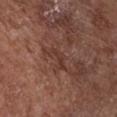This lesion was catalogued during total-body skin photography and was not selected for biopsy. A roughly 15 mm field-of-view crop from a total-body skin photograph. The total-body-photography lesion software estimated an area of roughly 3.5 mm² and an outline eccentricity of about 0.9 (0 = round, 1 = elongated). The lesion is located on the chest. A female subject aged 78 to 82. Approximately 3 mm at its widest.